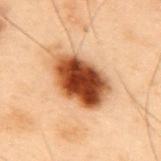diameter: ~6.5 mm (longest diameter)
patient: male, about 55 years old
acquisition: ~15 mm tile from a whole-body skin photo
location: the mid back